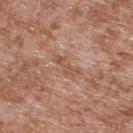The lesion is on the upper back. The lesion-visualizer software estimated a lesion area of about 3.5 mm², an outline eccentricity of about 0.9 (0 = round, 1 = elongated), and two-axis asymmetry of about 0.45. A male subject in their mid- to late 50s. The recorded lesion diameter is about 3 mm. A region of skin cropped from a whole-body photographic capture, roughly 15 mm wide.